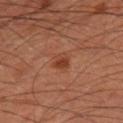Clinical impression: This lesion was catalogued during total-body skin photography and was not selected for biopsy.